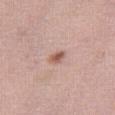Q: Was a biopsy performed?
A: catalogued during a skin exam; not biopsied
Q: What is the imaging modality?
A: ~15 mm crop, total-body skin-cancer survey
Q: Who is the patient?
A: female, aged 48–52
Q: Lesion location?
A: the right thigh